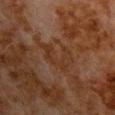follow-up = imaged on a skin check; not biopsied
tile lighting = cross-polarized
automated lesion analysis = a lesion area of about 8.5 mm², a shape eccentricity near 0.8, and two-axis asymmetry of about 0.5; a detector confidence of about 65 out of 100 that the crop contains a lesion
acquisition = total-body-photography crop, ~15 mm field of view
lesion diameter = ~4.5 mm (longest diameter)
patient = male, aged 78–82
body site = the chest